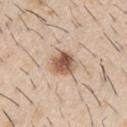Part of a total-body skin-imaging series; this lesion was reviewed on a skin check and was not flagged for biopsy. Longest diameter approximately 3.5 mm. A close-up tile cropped from a whole-body skin photograph, about 15 mm across. On the arm. The subject is a male aged approximately 60.